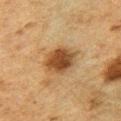No biopsy was performed on this lesion — it was imaged during a full skin examination and was not determined to be concerning.
A region of skin cropped from a whole-body photographic capture, roughly 15 mm wide.
A male subject approximately 50 years of age.
Approximately 4 mm at its widest.
On the left upper arm.
Captured under cross-polarized illumination.
An algorithmic analysis of the crop reported an area of roughly 11 mm², an eccentricity of roughly 0.3, and two-axis asymmetry of about 0.15. The analysis additionally found an automated nevus-likeness rating near 95 out of 100 and a detector confidence of about 100 out of 100 that the crop contains a lesion.